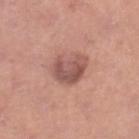The lesion was tiled from a total-body skin photograph and was not biopsied.
A male patient aged 28–32.
From the right lower leg.
Captured under white-light illumination.
The total-body-photography lesion software estimated a lesion color around L≈53 a*≈23 b*≈24 in CIELAB, roughly 11 lightness units darker than nearby skin, and a normalized lesion–skin contrast near 8. It also reported a border-irregularity rating of about 3.5/10, a within-lesion color-variation index near 4.5/10, and a peripheral color-asymmetry measure near 1.5.
Cropped from a total-body skin-imaging series; the visible field is about 15 mm.
The lesion's longest dimension is about 4 mm.